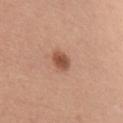Imaged during a routine full-body skin examination; the lesion was not biopsied and no histopathology is available.
A female subject in their 30s.
Located on the right upper arm.
Cropped from a whole-body photographic skin survey; the tile spans about 15 mm.